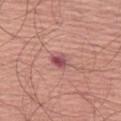Clinical impression: The lesion was tiled from a total-body skin photograph and was not biopsied. Clinical summary: The subject is a male aged approximately 65. Captured under white-light illumination. A 15 mm close-up extracted from a 3D total-body photography capture. The lesion is located on the left thigh.